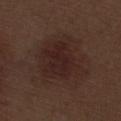follow-up: no biopsy performed (imaged during a skin exam)
subject: male, roughly 70 years of age
illumination: white-light
image: ~15 mm crop, total-body skin-cancer survey
body site: the right thigh
lesion diameter: about 6 mm
automated metrics: a mean CIELAB color near L≈24 a*≈17 b*≈19, about 6 CIELAB-L* units darker than the surrounding skin, and a lesion-to-skin contrast of about 7 (normalized; higher = more distinct)